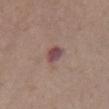Case summary:
- follow-up — imaged on a skin check; not biopsied
- subject — female, aged around 65
- imaging modality — total-body-photography crop, ~15 mm field of view
- automated lesion analysis — roughly 11 lightness units darker than nearby skin and a normalized lesion–skin contrast near 9.5; border irregularity of about 2 on a 0–10 scale, internal color variation of about 5 on a 0–10 scale, and a peripheral color-asymmetry measure near 2; a lesion-detection confidence of about 100/100
- site — the front of the torso
- lighting — white-light illumination